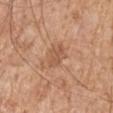Q: Was a biopsy performed?
A: catalogued during a skin exam; not biopsied
Q: Lesion size?
A: ≈3.5 mm
Q: What is the anatomic site?
A: the right upper arm
Q: Who is the patient?
A: male, roughly 65 years of age
Q: What kind of image is this?
A: 15 mm crop, total-body photography